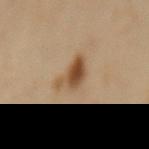follow-up = imaged on a skin check; not biopsied | site = the mid back | illumination = cross-polarized | patient = female, roughly 55 years of age | image = 15 mm crop, total-body photography | image-analysis metrics = an area of roughly 7 mm² and two-axis asymmetry of about 0.45; a lesion–skin lightness drop of about 13 and a normalized border contrast of about 9.5; border irregularity of about 4.5 on a 0–10 scale and radial color variation of about 2.